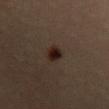| feature | finding |
|---|---|
| biopsy status | total-body-photography surveillance lesion; no biopsy |
| patient | female, roughly 30 years of age |
| lesion diameter | ≈3 mm |
| location | the mid back |
| tile lighting | cross-polarized illumination |
| imaging modality | 15 mm crop, total-body photography |